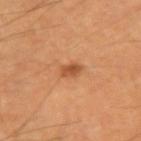<lesion>
  <biopsy_status>not biopsied; imaged during a skin examination</biopsy_status>
  <lesion_size>
    <long_diameter_mm_approx>2.0</long_diameter_mm_approx>
  </lesion_size>
  <site>left upper arm</site>
  <lighting>cross-polarized</lighting>
  <patient>
    <sex>male</sex>
    <age_approx>65</age_approx>
  </patient>
  <image>
    <source>total-body photography crop</source>
    <field_of_view_mm>15</field_of_view_mm>
  </image>
</lesion>